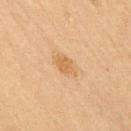Impression:
Captured during whole-body skin photography for melanoma surveillance; the lesion was not biopsied.
Image and clinical context:
Automated tile analysis of the lesion measured a footprint of about 5 mm² and two-axis asymmetry of about 0.2. It also reported a border-irregularity index near 2.5/10 and a peripheral color-asymmetry measure near 0.5. The software also gave a nevus-likeness score of about 65/100 and a detector confidence of about 100 out of 100 that the crop contains a lesion. Measured at roughly 3 mm in maximum diameter. A region of skin cropped from a whole-body photographic capture, roughly 15 mm wide. Captured under cross-polarized illumination. The patient is a male roughly 75 years of age. The lesion is located on the upper back.